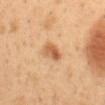The lesion was tiled from a total-body skin photograph and was not biopsied.
A close-up tile cropped from a whole-body skin photograph, about 15 mm across.
On the abdomen.
A male patient, aged 48 to 52.
About 3 mm across.
An algorithmic analysis of the crop reported a lesion area of about 5 mm², an outline eccentricity of about 0.75 (0 = round, 1 = elongated), and two-axis asymmetry of about 0.25. The analysis additionally found border irregularity of about 2 on a 0–10 scale and a peripheral color-asymmetry measure near 2.
Imaged with cross-polarized lighting.